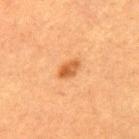Recorded during total-body skin imaging; not selected for excision or biopsy.
The lesion's longest dimension is about 2.5 mm.
This is a cross-polarized tile.
A region of skin cropped from a whole-body photographic capture, roughly 15 mm wide.
Automated tile analysis of the lesion measured border irregularity of about 2 on a 0–10 scale and a within-lesion color-variation index near 3/10. The analysis additionally found a classifier nevus-likeness of about 95/100 and a lesion-detection confidence of about 100/100.
The patient is a male aged around 50.
The lesion is on the mid back.